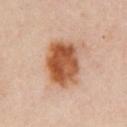follow-up=total-body-photography surveillance lesion; no biopsy
patient=approximately 55 years of age
anatomic site=the chest
diameter=about 5.5 mm
image=~15 mm crop, total-body skin-cancer survey
lighting=cross-polarized